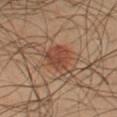Assessment: Imaged during a routine full-body skin examination; the lesion was not biopsied and no histopathology is available. Background: This is a cross-polarized tile. The total-body-photography lesion software estimated a mean CIELAB color near L≈32 a*≈17 b*≈23, a lesion–skin lightness drop of about 7, and a normalized lesion–skin contrast near 7. And it measured a border-irregularity index near 3.5/10. A 15 mm close-up tile from a total-body photography series done for melanoma screening. About 5 mm across. Located on the left forearm. A male patient, roughly 50 years of age.